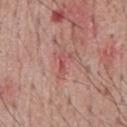This lesion was catalogued during total-body skin photography and was not selected for biopsy. A male subject, in their mid-60s. A 15 mm crop from a total-body photograph taken for skin-cancer surveillance. Automated image analysis of the tile measured an eccentricity of roughly 0.95 and a symmetry-axis asymmetry near 0.4. And it measured a mean CIELAB color near L≈53 a*≈28 b*≈25 and a normalized lesion–skin contrast near 5.5. The software also gave a border-irregularity rating of about 5.5/10, internal color variation of about 0 on a 0–10 scale, and a peripheral color-asymmetry measure near 0. It also reported a classifier nevus-likeness of about 0/100 and a detector confidence of about 90 out of 100 that the crop contains a lesion. The recorded lesion diameter is about 3.5 mm. Located on the chest. The tile uses white-light illumination.